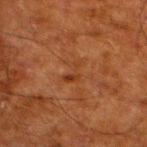The lesion is located on the left lower leg. Cropped from a total-body skin-imaging series; the visible field is about 15 mm. The subject is a male in their 80s.